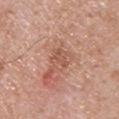tile lighting=white-light; acquisition=~15 mm tile from a whole-body skin photo; patient=male, aged 68 to 72; anatomic site=the upper back.